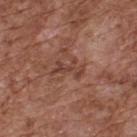Case summary:
• workup · no biopsy performed (imaged during a skin exam)